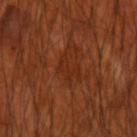The lesion was photographed on a routine skin check and not biopsied; there is no pathology result. A lesion tile, about 15 mm wide, cut from a 3D total-body photograph. The lesion is on the right upper arm. A male patient, approximately 70 years of age.